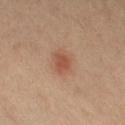- workup — catalogued during a skin exam; not biopsied
- illumination — cross-polarized
- anatomic site — the left leg
- patient — female, about 40 years old
- acquisition — ~15 mm tile from a whole-body skin photo
- size — ≈3 mm
- TBP lesion metrics — a color-variation rating of about 2.5/10 and a peripheral color-asymmetry measure near 1; a classifier nevus-likeness of about 90/100